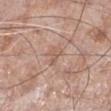A male patient, aged approximately 75.
Cropped from a whole-body photographic skin survey; the tile spans about 15 mm.
The lesion is on the right lower leg.
This is a white-light tile.